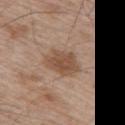<lesion>
  <biopsy_status>not biopsied; imaged during a skin examination</biopsy_status>
  <image>
    <source>total-body photography crop</source>
    <field_of_view_mm>15</field_of_view_mm>
  </image>
  <lighting>white-light</lighting>
  <site>upper back</site>
  <patient>
    <sex>male</sex>
    <age_approx>65</age_approx>
  </patient>
</lesion>